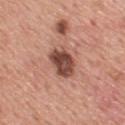biopsy_status: not biopsied; imaged during a skin examination
site: upper back
automated_metrics:
  vs_skin_darker_L: 16.0
image:
  source: total-body photography crop
  field_of_view_mm: 15
lesion_size:
  long_diameter_mm_approx: 3.5
patient:
  sex: male
  age_approx: 60
lighting: white-light